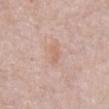* notes: catalogued during a skin exam; not biopsied
* lesion size: ~2.5 mm (longest diameter)
* anatomic site: the abdomen
* patient: male, aged 78 to 82
* image source: ~15 mm crop, total-body skin-cancer survey